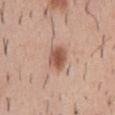- biopsy status — catalogued during a skin exam; not biopsied
- tile lighting — white-light illumination
- imaging modality — 15 mm crop, total-body photography
- subject — male, aged around 40
- body site — the chest